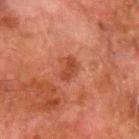Recorded during total-body skin imaging; not selected for excision or biopsy.
A male patient aged 78–82.
Measured at roughly 3 mm in maximum diameter.
An algorithmic analysis of the crop reported about 7 CIELAB-L* units darker than the surrounding skin and a normalized lesion–skin contrast near 7. It also reported a classifier nevus-likeness of about 5/100 and a lesion-detection confidence of about 100/100.
The lesion is on the left forearm.
A 15 mm close-up extracted from a 3D total-body photography capture.